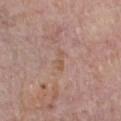{"biopsy_status": "not biopsied; imaged during a skin examination", "lesion_size": {"long_diameter_mm_approx": 2.5}, "patient": {"sex": "male", "age_approx": 75}, "image": {"source": "total-body photography crop", "field_of_view_mm": 15}, "site": "chest", "lighting": "white-light", "automated_metrics": {"cielab_L": 55, "cielab_a": 18, "cielab_b": 28, "vs_skin_darker_L": 6.0, "vs_skin_contrast_norm": 6.0, "border_irregularity_0_10": 4.5, "color_variation_0_10": 0.0, "peripheral_color_asymmetry": 0.0, "nevus_likeness_0_100": 0, "lesion_detection_confidence_0_100": 100}}